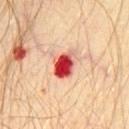image source: ~15 mm tile from a whole-body skin photo
lesion size: ≈3.5 mm
tile lighting: cross-polarized
subject: male, aged approximately 30
body site: the front of the torso